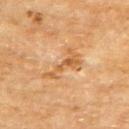Imaged during a routine full-body skin examination; the lesion was not biopsied and no histopathology is available. The lesion is on the upper back. The recorded lesion diameter is about 5 mm. A male patient, approximately 65 years of age. A 15 mm close-up extracted from a 3D total-body photography capture. This is a cross-polarized tile.